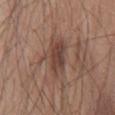Assessment:
The lesion was tiled from a total-body skin photograph and was not biopsied.
Image and clinical context:
From the front of the torso. The recorded lesion diameter is about 4.5 mm. A male patient aged 53 to 57. A 15 mm crop from a total-body photograph taken for skin-cancer surveillance. Automated image analysis of the tile measured a nevus-likeness score of about 5/100 and lesion-presence confidence of about 100/100.